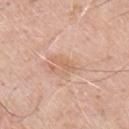Recorded during total-body skin imaging; not selected for excision or biopsy. From the chest. A male patient aged 68 to 72. Approximately 3 mm at its widest. An algorithmic analysis of the crop reported border irregularity of about 3.5 on a 0–10 scale, a color-variation rating of about 0/10, and radial color variation of about 0. And it measured a nevus-likeness score of about 0/100 and a lesion-detection confidence of about 100/100. This image is a 15 mm lesion crop taken from a total-body photograph.